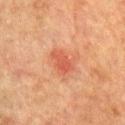biopsy_status: not biopsied; imaged during a skin examination
lesion_size:
  long_diameter_mm_approx: 3.5
lighting: cross-polarized
patient:
  sex: male
  age_approx: 75
image:
  source: total-body photography crop
  field_of_view_mm: 15
site: right forearm